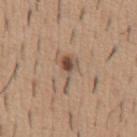workup=total-body-photography surveillance lesion; no biopsy
image=total-body-photography crop, ~15 mm field of view
diameter=~4.5 mm (longest diameter)
tile lighting=white-light
subject=male, roughly 40 years of age
site=the front of the torso
TBP lesion metrics=a mean CIELAB color near L≈51 a*≈16 b*≈27 and about 12 CIELAB-L* units darker than the surrounding skin